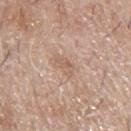Assessment: Captured during whole-body skin photography for melanoma surveillance; the lesion was not biopsied. Context: Located on the upper back. A male patient in their mid- to late 50s. Automated tile analysis of the lesion measured a footprint of about 3 mm², a shape eccentricity near 0.9, and a shape-asymmetry score of about 0.5 (0 = symmetric). It also reported a border-irregularity index near 6/10, a color-variation rating of about 0.5/10, and radial color variation of about 0. And it measured a classifier nevus-likeness of about 0/100 and a lesion-detection confidence of about 100/100. Cropped from a whole-body photographic skin survey; the tile spans about 15 mm.